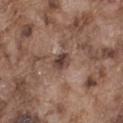Q: Automated lesion metrics?
A: an area of roughly 4 mm² and two-axis asymmetry of about 0.25; a nevus-likeness score of about 20/100 and lesion-presence confidence of about 100/100
Q: Lesion location?
A: the leg
Q: How large is the lesion?
A: about 2.5 mm
Q: Illumination type?
A: white-light illumination
Q: Who is the patient?
A: male, aged 73 to 77
Q: How was this image acquired?
A: ~15 mm tile from a whole-body skin photo